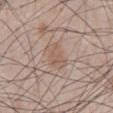Findings:
• workup: imaged on a skin check; not biopsied
• diameter: ≈3.5 mm
• location: the abdomen
• image: ~15 mm tile from a whole-body skin photo
• patient: male, aged 48 to 52